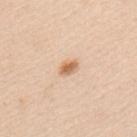Q: Was this lesion biopsied?
A: catalogued during a skin exam; not biopsied
Q: How was the tile lit?
A: white-light illumination
Q: Patient demographics?
A: female, approximately 45 years of age
Q: What is the imaging modality?
A: total-body-photography crop, ~15 mm field of view
Q: Lesion size?
A: ~2.5 mm (longest diameter)
Q: Where on the body is the lesion?
A: the back